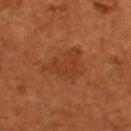This lesion was catalogued during total-body skin photography and was not selected for biopsy.
Imaged with cross-polarized lighting.
A roughly 15 mm field-of-view crop from a total-body skin photograph.
The lesion-visualizer software estimated a footprint of about 13 mm², a shape eccentricity near 0.75, and two-axis asymmetry of about 0.45. It also reported a lesion color around L≈36 a*≈25 b*≈33 in CIELAB, about 6 CIELAB-L* units darker than the surrounding skin, and a normalized lesion–skin contrast near 5. And it measured border irregularity of about 5 on a 0–10 scale and a peripheral color-asymmetry measure near 0.5. The analysis additionally found a nevus-likeness score of about 0/100 and lesion-presence confidence of about 100/100.
A male patient aged 53–57.
Located on the upper back.